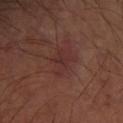Clinical impression: No biopsy was performed on this lesion — it was imaged during a full skin examination and was not determined to be concerning. Background: The lesion-visualizer software estimated a border-irregularity index near 5.5/10, a within-lesion color-variation index near 1/10, and peripheral color asymmetry of about 0. A male subject aged 48–52. This image is a 15 mm lesion crop taken from a total-body photograph. Imaged with cross-polarized lighting. From the left arm. About 3 mm across.